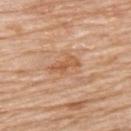A female subject, aged 73–77.
This is a white-light tile.
The lesion is located on the upper back.
A 15 mm close-up tile from a total-body photography series done for melanoma screening.